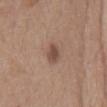Recorded during total-body skin imaging; not selected for excision or biopsy. The lesion's longest dimension is about 2.5 mm. A 15 mm close-up extracted from a 3D total-body photography capture. Captured under white-light illumination. An algorithmic analysis of the crop reported a lesion color around L≈48 a*≈18 b*≈25 in CIELAB and a lesion–skin lightness drop of about 10. The lesion is located on the chest. The patient is a male approximately 75 years of age.